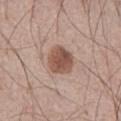<lesion>
<biopsy_status>not biopsied; imaged during a skin examination</biopsy_status>
<site>front of the torso</site>
<image>
  <source>total-body photography crop</source>
  <field_of_view_mm>15</field_of_view_mm>
</image>
<lighting>white-light</lighting>
<patient>
  <sex>male</sex>
  <age_approx>65</age_approx>
</patient>
<automated_metrics>
  <cielab_L>52</cielab_L>
  <cielab_a>20</cielab_a>
  <cielab_b>26</cielab_b>
  <vs_skin_darker_L>13.0</vs_skin_darker_L>
  <vs_skin_contrast_norm>9.0</vs_skin_contrast_norm>
</automated_metrics>
</lesion>